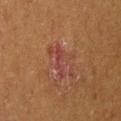Recorded during total-body skin imaging; not selected for excision or biopsy. A female patient approximately 50 years of age. Located on the right thigh. Automated image analysis of the tile measured a lesion area of about 7 mm², an eccentricity of roughly 0.85, and two-axis asymmetry of about 0.55. The software also gave a border-irregularity index near 7.5/10. Longest diameter approximately 4.5 mm. A close-up tile cropped from a whole-body skin photograph, about 15 mm across.